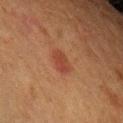Assessment:
The lesion was tiled from a total-body skin photograph and was not biopsied.
Clinical summary:
The recorded lesion diameter is about 3 mm. Located on the left upper arm. Imaged with cross-polarized lighting. A 15 mm close-up extracted from a 3D total-body photography capture. The patient is a female aged 48 to 52.